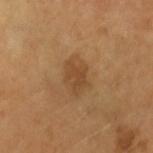This lesion was catalogued during total-body skin photography and was not selected for biopsy.
A lesion tile, about 15 mm wide, cut from a 3D total-body photograph.
The lesion is located on the left forearm.
Imaged with cross-polarized lighting.
The patient is a female in their mid-50s.
Approximately 4 mm at its widest.